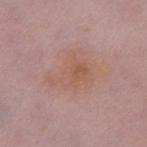The lesion was photographed on a routine skin check and not biopsied; there is no pathology result. Captured under white-light illumination. Cropped from a total-body skin-imaging series; the visible field is about 15 mm. The lesion is on the chest. The recorded lesion diameter is about 5.5 mm. A female subject aged approximately 50.